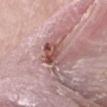Recorded during total-body skin imaging; not selected for excision or biopsy. A close-up tile cropped from a whole-body skin photograph, about 15 mm across. On the left forearm. The subject is a male aged 63 to 67.